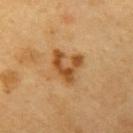Recorded during total-body skin imaging; not selected for excision or biopsy. The patient is a male about 60 years old. The lesion is on the arm. A 15 mm crop from a total-body photograph taken for skin-cancer surveillance. Automated image analysis of the tile measured an area of roughly 8.5 mm², an outline eccentricity of about 0.5 (0 = round, 1 = elongated), and a shape-asymmetry score of about 0.6 (0 = symmetric). And it measured a lesion color around L≈50 a*≈22 b*≈42 in CIELAB and a lesion-to-skin contrast of about 9 (normalized; higher = more distinct).